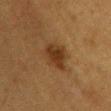follow-up = no biopsy performed (imaged during a skin exam); location = the chest; subject = female, aged 38–42; image source = 15 mm crop, total-body photography.